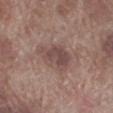Acquisition and patient details: The patient is a male roughly 70 years of age. Automated tile analysis of the lesion measured a border-irregularity rating of about 3/10 and a color-variation rating of about 3/10. And it measured a classifier nevus-likeness of about 0/100 and a lesion-detection confidence of about 100/100. A region of skin cropped from a whole-body photographic capture, roughly 15 mm wide. Imaged with white-light lighting. Approximately 4.5 mm at its widest. From the leg.